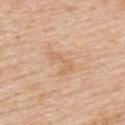Q: Was this lesion biopsied?
A: total-body-photography surveillance lesion; no biopsy
Q: What is the anatomic site?
A: the upper back
Q: Lesion size?
A: about 3.5 mm
Q: How was the tile lit?
A: white-light illumination
Q: What is the imaging modality?
A: ~15 mm crop, total-body skin-cancer survey
Q: Patient demographics?
A: male, aged 58–62